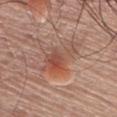Clinical impression: Imaged during a routine full-body skin examination; the lesion was not biopsied and no histopathology is available. Clinical summary: Cropped from a total-body skin-imaging series; the visible field is about 15 mm. An algorithmic analysis of the crop reported an area of roughly 11 mm² and a shape-asymmetry score of about 0.45 (0 = symmetric). The software also gave a lesion-to-skin contrast of about 6.5 (normalized; higher = more distinct). The software also gave a border-irregularity index near 7/10 and a peripheral color-asymmetry measure near 2. Approximately 5.5 mm at its widest. On the front of the torso. A male subject in their 60s.